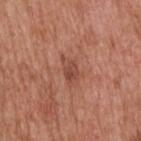The lesion was photographed on a routine skin check and not biopsied; there is no pathology result.
Measured at roughly 3.5 mm in maximum diameter.
Cropped from a whole-body photographic skin survey; the tile spans about 15 mm.
Located on the head or neck.
A male subject, roughly 60 years of age.